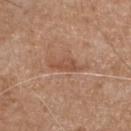Part of a total-body skin-imaging series; this lesion was reviewed on a skin check and was not flagged for biopsy.
The patient is a male aged 68–72.
This image is a 15 mm lesion crop taken from a total-body photograph.
Longest diameter approximately 3.5 mm.
Automated image analysis of the tile measured a mean CIELAB color near L≈51 a*≈22 b*≈32, about 8 CIELAB-L* units darker than the surrounding skin, and a normalized lesion–skin contrast near 6. It also reported internal color variation of about 1.5 on a 0–10 scale and radial color variation of about 0.5.
On the chest.
Imaged with white-light lighting.